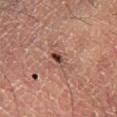Q: Is there a histopathology result?
A: total-body-photography surveillance lesion; no biopsy
Q: Who is the patient?
A: male, in their mid- to late 50s
Q: What is the lesion's diameter?
A: ~2.5 mm (longest diameter)
Q: How was this image acquired?
A: ~15 mm crop, total-body skin-cancer survey
Q: How was the tile lit?
A: cross-polarized
Q: Where on the body is the lesion?
A: the right lower leg